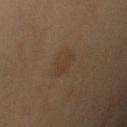Q: Is there a histopathology result?
A: total-body-photography surveillance lesion; no biopsy
Q: Who is the patient?
A: male, aged approximately 60
Q: What is the lesion's diameter?
A: about 3.5 mm
Q: What kind of image is this?
A: 15 mm crop, total-body photography
Q: What did automated image analysis measure?
A: a lesion–skin lightness drop of about 4 and a normalized border contrast of about 4.5; a border-irregularity index near 2.5/10 and a color-variation rating of about 1.5/10; a classifier nevus-likeness of about 50/100
Q: Lesion location?
A: the left upper arm
Q: Illumination type?
A: cross-polarized illumination